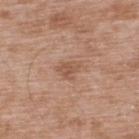follow-up — catalogued during a skin exam; not biopsied
subject — male, aged approximately 50
site — the back
imaging modality — total-body-photography crop, ~15 mm field of view